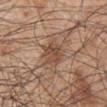{"biopsy_status": "not biopsied; imaged during a skin examination", "lighting": "white-light", "image": {"source": "total-body photography crop", "field_of_view_mm": 15}, "patient": {"sex": "male", "age_approx": 45}, "site": "right upper arm"}